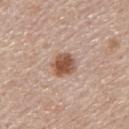<record>
<biopsy_status>not biopsied; imaged during a skin examination</biopsy_status>
<patient>
  <sex>male</sex>
  <age_approx>60</age_approx>
</patient>
<site>upper back</site>
<image>
  <source>total-body photography crop</source>
  <field_of_view_mm>15</field_of_view_mm>
</image>
</record>